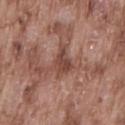No biopsy was performed on this lesion — it was imaged during a full skin examination and was not determined to be concerning.
Approximately 3.5 mm at its widest.
A region of skin cropped from a whole-body photographic capture, roughly 15 mm wide.
A male subject about 75 years old.
Imaged with white-light lighting.
Located on the lower back.
Automated image analysis of the tile measured an area of roughly 6 mm² and a shape-asymmetry score of about 0.45 (0 = symmetric). The analysis additionally found a mean CIELAB color near L≈45 a*≈22 b*≈25 and a lesion–skin lightness drop of about 10. And it measured a border-irregularity rating of about 5/10 and radial color variation of about 1.